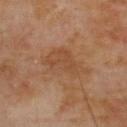Longest diameter approximately 6.5 mm.
The lesion is located on the upper back.
A 15 mm close-up extracted from a 3D total-body photography capture.
The total-body-photography lesion software estimated a lesion area of about 14 mm², an outline eccentricity of about 0.9 (0 = round, 1 = elongated), and a shape-asymmetry score of about 0.3 (0 = symmetric). The analysis additionally found a lesion color around L≈47 a*≈20 b*≈33 in CIELAB, roughly 6 lightness units darker than nearby skin, and a normalized lesion–skin contrast near 5.5. The analysis additionally found a nevus-likeness score of about 0/100.
The subject is a male about 70 years old.
This is a cross-polarized tile.